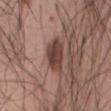Assessment:
This lesion was catalogued during total-body skin photography and was not selected for biopsy.
Image and clinical context:
The tile uses white-light illumination. On the abdomen. About 4.5 mm across. A 15 mm close-up tile from a total-body photography series done for melanoma screening. A male patient in their mid- to late 50s.